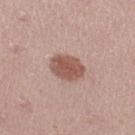Findings:
* notes: no biopsy performed (imaged during a skin exam)
* imaging modality: ~15 mm tile from a whole-body skin photo
* anatomic site: the right thigh
* illumination: white-light
* diameter: ~3.5 mm (longest diameter)
* patient: female, roughly 20 years of age